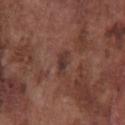Assessment:
Captured during whole-body skin photography for melanoma surveillance; the lesion was not biopsied.
Context:
A male subject in their mid-70s. The total-body-photography lesion software estimated a lesion area of about 3 mm² and a symmetry-axis asymmetry near 0.3. It also reported a border-irregularity index near 3.5/10 and a peripheral color-asymmetry measure near 0. The analysis additionally found a nevus-likeness score of about 0/100. A region of skin cropped from a whole-body photographic capture, roughly 15 mm wide. The tile uses white-light illumination. On the chest. Measured at roughly 3 mm in maximum diameter.